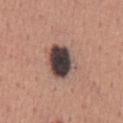Captured during whole-body skin photography for melanoma surveillance; the lesion was not biopsied.
Measured at roughly 5 mm in maximum diameter.
The subject is a male aged approximately 45.
This is a white-light tile.
A close-up tile cropped from a whole-body skin photograph, about 15 mm across.
On the chest.